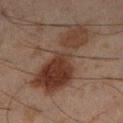Recorded during total-body skin imaging; not selected for excision or biopsy.